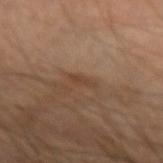Findings:
- follow-up — no biopsy performed (imaged during a skin exam)
- patient — male, aged 68 to 72
- automated metrics — a lesion color around L≈40 a*≈17 b*≈29 in CIELAB and a normalized border contrast of about 6
- image — ~15 mm tile from a whole-body skin photo
- tile lighting — cross-polarized
- diameter — about 2.5 mm
- body site — the left forearm